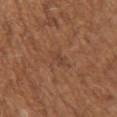No biopsy was performed on this lesion — it was imaged during a full skin examination and was not determined to be concerning.
This image is a 15 mm lesion crop taken from a total-body photograph.
On the left upper arm.
The lesion's longest dimension is about 3 mm.
The subject is a female in their mid- to late 70s.
Captured under white-light illumination.